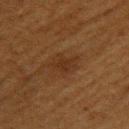<tbp_lesion>
<biopsy_status>not biopsied; imaged during a skin examination</biopsy_status>
<patient>
  <sex>female</sex>
  <age_approx>40</age_approx>
</patient>
<site>arm</site>
<lesion_size>
  <long_diameter_mm_approx>2.5</long_diameter_mm_approx>
</lesion_size>
<lighting>cross-polarized</lighting>
<automated_metrics>
  <eccentricity>0.75</eccentricity>
  <shape_asymmetry>0.2</shape_asymmetry>
  <cielab_L>25</cielab_L>
  <cielab_a>17</cielab_a>
  <cielab_b>26</cielab_b>
  <vs_skin_darker_L>5.0</vs_skin_darker_L>
  <vs_skin_contrast_norm>6.0</vs_skin_contrast_norm>
  <nevus_likeness_0_100>10</nevus_likeness_0_100>
</automated_metrics>
<image>
  <source>total-body photography crop</source>
  <field_of_view_mm>15</field_of_view_mm>
</image>
</tbp_lesion>